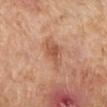| field | value |
|---|---|
| biopsy status | total-body-photography surveillance lesion; no biopsy |
| location | the right lower leg |
| lighting | cross-polarized |
| lesion size | ≈4 mm |
| automated metrics | a mean CIELAB color near L≈56 a*≈23 b*≈33, a lesion–skin lightness drop of about 9, and a normalized lesion–skin contrast near 6; a color-variation rating of about 3.5/10 |
| imaging modality | total-body-photography crop, ~15 mm field of view |
| patient | female, in their mid- to late 50s |